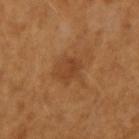The patient is a female aged 53–57.
A 15 mm close-up tile from a total-body photography series done for melanoma screening.
The lesion is on the right forearm.